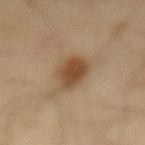Captured during whole-body skin photography for melanoma surveillance; the lesion was not biopsied.
A male patient, aged 38 to 42.
The total-body-photography lesion software estimated a lesion color around L≈47 a*≈17 b*≈33 in CIELAB, about 12 CIELAB-L* units darker than the surrounding skin, and a normalized border contrast of about 9.
Captured under cross-polarized illumination.
Longest diameter approximately 4 mm.
From the mid back.
This image is a 15 mm lesion crop taken from a total-body photograph.